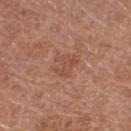| feature | finding |
|---|---|
| TBP lesion metrics | a lesion area of about 5 mm² and an outline eccentricity of about 0.5 (0 = round, 1 = elongated); an automated nevus-likeness rating near 0 out of 100 and lesion-presence confidence of about 100/100 |
| site | the leg |
| patient | female, aged around 55 |
| diameter | ~2.5 mm (longest diameter) |
| lighting | white-light illumination |
| image | total-body-photography crop, ~15 mm field of view |